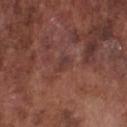Background:
From the front of the torso. Longest diameter approximately 2.5 mm. An algorithmic analysis of the crop reported an outline eccentricity of about 0.85 (0 = round, 1 = elongated) and two-axis asymmetry of about 0.35. The software also gave an average lesion color of about L≈37 a*≈21 b*≈22 (CIELAB), roughly 5 lightness units darker than nearby skin, and a normalized lesion–skin contrast near 5. The software also gave a classifier nevus-likeness of about 0/100. A 15 mm close-up extracted from a 3D total-body photography capture. A male subject in their mid-70s. Captured under white-light illumination.